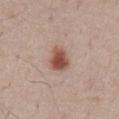The lesion was photographed on a routine skin check and not biopsied; there is no pathology result. Automated tile analysis of the lesion measured a mean CIELAB color near L≈52 a*≈22 b*≈26, about 14 CIELAB-L* units darker than the surrounding skin, and a lesion-to-skin contrast of about 9.5 (normalized; higher = more distinct). It also reported a border-irregularity rating of about 2/10, internal color variation of about 4.5 on a 0–10 scale, and peripheral color asymmetry of about 1. The analysis additionally found a lesion-detection confidence of about 100/100. On the abdomen. The tile uses white-light illumination. The patient is a male aged 53 to 57. About 3.5 mm across. A region of skin cropped from a whole-body photographic capture, roughly 15 mm wide.